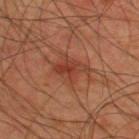Imaged during a routine full-body skin examination; the lesion was not biopsied and no histopathology is available. The patient is a male in their mid- to late 40s. A close-up tile cropped from a whole-body skin photograph, about 15 mm across. Imaged with cross-polarized lighting. On the upper back. Measured at roughly 4 mm in maximum diameter. Automated image analysis of the tile measured a footprint of about 8.5 mm², an outline eccentricity of about 0.7 (0 = round, 1 = elongated), and a symmetry-axis asymmetry near 0.3. The software also gave a mean CIELAB color near L≈39 a*≈25 b*≈30, about 7 CIELAB-L* units darker than the surrounding skin, and a lesion-to-skin contrast of about 6.5 (normalized; higher = more distinct). The software also gave a classifier nevus-likeness of about 35/100 and a lesion-detection confidence of about 100/100.